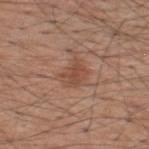biopsy_status: not biopsied; imaged during a skin examination
patient:
  sex: male
  age_approx: 60
image:
  source: total-body photography crop
  field_of_view_mm: 15
site: upper back
lesion_size:
  long_diameter_mm_approx: 3.0
lighting: white-light
automated_metrics:
  area_mm2_approx: 5.5
  shape_asymmetry: 0.35
  border_irregularity_0_10: 3.5
  color_variation_0_10: 2.0
  peripheral_color_asymmetry: 0.5
  lesion_detection_confidence_0_100: 100